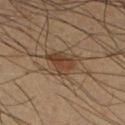{"biopsy_status": "not biopsied; imaged during a skin examination", "patient": {"sex": "male", "age_approx": 40}, "image": {"source": "total-body photography crop", "field_of_view_mm": 15}, "lighting": "cross-polarized", "site": "leg", "lesion_size": {"long_diameter_mm_approx": 4.0}}